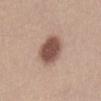{
  "biopsy_status": "not biopsied; imaged during a skin examination"
}